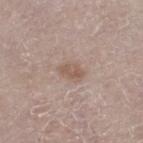Assessment: Imaged during a routine full-body skin examination; the lesion was not biopsied and no histopathology is available. Background: Imaged with white-light lighting. The lesion's longest dimension is about 3 mm. A male subject, aged 78–82. A close-up tile cropped from a whole-body skin photograph, about 15 mm across. Located on the leg.